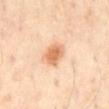workup = imaged on a skin check; not biopsied | patient = male, roughly 65 years of age | image source = 15 mm crop, total-body photography | lighting = cross-polarized | anatomic site = the abdomen.